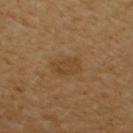Captured during whole-body skin photography for melanoma surveillance; the lesion was not biopsied. A female patient, about 55 years old. Imaged with cross-polarized lighting. Cropped from a whole-body photographic skin survey; the tile spans about 15 mm. The lesion's longest dimension is about 3.5 mm. From the chest.